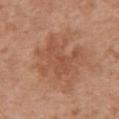Imaged during a routine full-body skin examination; the lesion was not biopsied and no histopathology is available. This image is a 15 mm lesion crop taken from a total-body photograph. The patient is a female aged around 65. The lesion is on the chest. Measured at roughly 5.5 mm in maximum diameter.